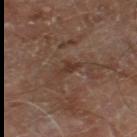<tbp_lesion>
  <biopsy_status>not biopsied; imaged during a skin examination</biopsy_status>
  <image>
    <source>total-body photography crop</source>
    <field_of_view_mm>15</field_of_view_mm>
  </image>
  <lesion_size>
    <long_diameter_mm_approx>2.5</long_diameter_mm_approx>
  </lesion_size>
  <patient>
    <sex>male</sex>
    <age_approx>70</age_approx>
  </patient>
  <site>left lower leg</site>
</tbp_lesion>